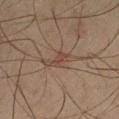Acquisition and patient details:
A 15 mm close-up tile from a total-body photography series done for melanoma screening. The recorded lesion diameter is about 4 mm. A male patient, aged approximately 45. The tile uses cross-polarized illumination. The lesion is located on the left thigh.